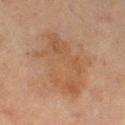Q: Was a biopsy performed?
A: no biopsy performed (imaged during a skin exam)
Q: What is the anatomic site?
A: the right thigh
Q: What is the imaging modality?
A: 15 mm crop, total-body photography
Q: Lesion size?
A: ~8 mm (longest diameter)
Q: What are the patient's age and sex?
A: female, aged 68 to 72